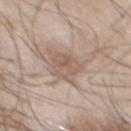| key | value |
|---|---|
| patient | male, aged around 55 |
| automated metrics | internal color variation of about 4 on a 0–10 scale and peripheral color asymmetry of about 1.5 |
| acquisition | total-body-photography crop, ~15 mm field of view |
| size | ≈4 mm |
| illumination | white-light illumination |
| anatomic site | the chest |